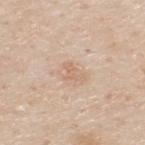Context:
Imaged with white-light lighting. Located on the upper back. The patient is a male roughly 80 years of age. A roughly 15 mm field-of-view crop from a total-body skin photograph. Measured at roughly 2.5 mm in maximum diameter.